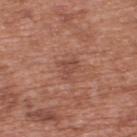<case>
<biopsy_status>not biopsied; imaged during a skin examination</biopsy_status>
<lighting>white-light</lighting>
<image>
  <source>total-body photography crop</source>
  <field_of_view_mm>15</field_of_view_mm>
</image>
<site>upper back</site>
<patient>
  <sex>male</sex>
  <age_approx>70</age_approx>
</patient>
<lesion_size>
  <long_diameter_mm_approx>2.5</long_diameter_mm_approx>
</lesion_size>
</case>